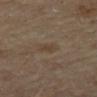A close-up tile cropped from a whole-body skin photograph, about 15 mm across.
On the upper back.
Imaged with cross-polarized lighting.
A female patient, roughly 60 years of age.
The recorded lesion diameter is about 2.5 mm.
The total-body-photography lesion software estimated an area of roughly 2.5 mm², an eccentricity of roughly 0.9, and a shape-asymmetry score of about 0.25 (0 = symmetric). And it measured an average lesion color of about L≈39 a*≈13 b*≈26 (CIELAB), about 5 CIELAB-L* units darker than the surrounding skin, and a normalized border contrast of about 5.5. The analysis additionally found a border-irregularity index near 2.5/10.